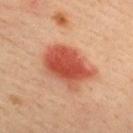Q: Was a biopsy performed?
A: catalogued during a skin exam; not biopsied
Q: Where on the body is the lesion?
A: the upper back
Q: How was the tile lit?
A: cross-polarized illumination
Q: Patient demographics?
A: male, approximately 40 years of age
Q: Automated lesion metrics?
A: a lesion area of about 20 mm²; an average lesion color of about L≈47 a*≈30 b*≈31 (CIELAB) and a normalized lesion–skin contrast near 10; a border-irregularity rating of about 2.5/10, a color-variation rating of about 6/10, and a peripheral color-asymmetry measure near 2
Q: What is the imaging modality?
A: total-body-photography crop, ~15 mm field of view
Q: How large is the lesion?
A: ~6.5 mm (longest diameter)